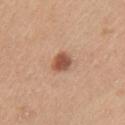Clinical impression: The lesion was photographed on a routine skin check and not biopsied; there is no pathology result. Acquisition and patient details: Automated image analysis of the tile measured an area of roughly 5 mm², an outline eccentricity of about 0.5 (0 = round, 1 = elongated), and two-axis asymmetry of about 0.15. It also reported a peripheral color-asymmetry measure near 1.5. A female subject roughly 45 years of age. Captured under white-light illumination. Cropped from a total-body skin-imaging series; the visible field is about 15 mm. The lesion is located on the left upper arm.